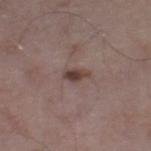– notes — no biopsy performed (imaged during a skin exam)
– location — the right lower leg
– lesion diameter — ~2.5 mm (longest diameter)
– lighting — white-light
– patient — male, in their mid- to late 50s
– TBP lesion metrics — a lesion color around L≈40 a*≈17 b*≈19 in CIELAB and a normalized border contrast of about 9.5; internal color variation of about 2 on a 0–10 scale and radial color variation of about 0.5; a nevus-likeness score of about 85/100 and a lesion-detection confidence of about 100/100
– imaging modality — 15 mm crop, total-body photography